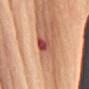biopsy status — catalogued during a skin exam; not biopsied | patient — female, aged 63 to 67 | site — the left arm | image-analysis metrics — a lesion color around L≈51 a*≈32 b*≈29 in CIELAB and roughly 13 lightness units darker than nearby skin; a color-variation rating of about 10/10 and peripheral color asymmetry of about 4 | image — ~15 mm tile from a whole-body skin photo.